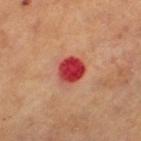notes: imaged on a skin check; not biopsied | lighting: cross-polarized | image-analysis metrics: a footprint of about 8.5 mm², an outline eccentricity of about 0.5 (0 = round, 1 = elongated), and a symmetry-axis asymmetry near 0.15; a mean CIELAB color near L≈47 a*≈43 b*≈32, roughly 17 lightness units darker than nearby skin, and a lesion-to-skin contrast of about 11.5 (normalized; higher = more distinct); border irregularity of about 1.5 on a 0–10 scale, internal color variation of about 6.5 on a 0–10 scale, and a peripheral color-asymmetry measure near 2 | acquisition: total-body-photography crop, ~15 mm field of view | location: the left thigh | patient: female, aged approximately 60.